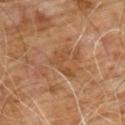Notes:
* biopsy status: total-body-photography surveillance lesion; no biopsy
* site: the chest
* imaging modality: ~15 mm tile from a whole-body skin photo
* patient: male, roughly 60 years of age